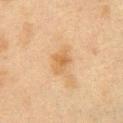Clinical impression: Captured during whole-body skin photography for melanoma surveillance; the lesion was not biopsied. Clinical summary: A close-up tile cropped from a whole-body skin photograph, about 15 mm across. A female patient about 40 years old. The lesion is located on the right upper arm. The recorded lesion diameter is about 2.5 mm. The total-body-photography lesion software estimated an area of roughly 4 mm² and a shape-asymmetry score of about 0.3 (0 = symmetric). The software also gave a lesion color around L≈52 a*≈17 b*≈36 in CIELAB, a lesion–skin lightness drop of about 7, and a lesion-to-skin contrast of about 6.5 (normalized; higher = more distinct). The software also gave border irregularity of about 3 on a 0–10 scale, internal color variation of about 3 on a 0–10 scale, and radial color variation of about 1. The analysis additionally found an automated nevus-likeness rating near 5 out of 100 and lesion-presence confidence of about 100/100.